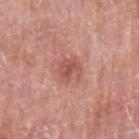{
  "biopsy_status": "not biopsied; imaged during a skin examination",
  "patient": {
    "sex": "female",
    "age_approx": 60
  },
  "site": "left upper arm",
  "image": {
    "source": "total-body photography crop",
    "field_of_view_mm": 15
  },
  "automated_metrics": {
    "cielab_L": 54,
    "cielab_a": 27,
    "cielab_b": 27,
    "vs_skin_darker_L": 9.0,
    "vs_skin_contrast_norm": 6.5,
    "border_irregularity_0_10": 3.5,
    "color_variation_0_10": 2.5,
    "peripheral_color_asymmetry": 0.5
  }
}